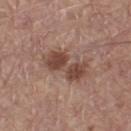Q: Was a biopsy performed?
A: total-body-photography surveillance lesion; no biopsy
Q: Lesion location?
A: the left thigh
Q: How was this image acquired?
A: ~15 mm tile from a whole-body skin photo
Q: Patient demographics?
A: male, approximately 65 years of age